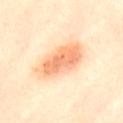Notes:
* notes — imaged on a skin check; not biopsied
* size — ~6 mm (longest diameter)
* body site — the abdomen
* acquisition — 15 mm crop, total-body photography
* illumination — cross-polarized illumination
* patient — female, aged around 60
* automated lesion analysis — an automated nevus-likeness rating near 95 out of 100 and a detector confidence of about 100 out of 100 that the crop contains a lesion